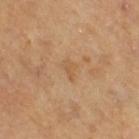This lesion was catalogued during total-body skin photography and was not selected for biopsy. The tile uses cross-polarized illumination. The patient is a female approximately 55 years of age. Approximately 2.5 mm at its widest. The lesion is located on the right thigh. A close-up tile cropped from a whole-body skin photograph, about 15 mm across.